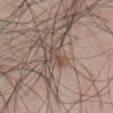No biopsy was performed on this lesion — it was imaged during a full skin examination and was not determined to be concerning. The lesion's longest dimension is about 3 mm. This is a white-light tile. A close-up tile cropped from a whole-body skin photograph, about 15 mm across. A male subject, approximately 45 years of age. Located on the abdomen.